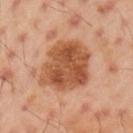workup — no biopsy performed (imaged during a skin exam)
lighting — cross-polarized
diameter — ~6 mm (longest diameter)
automated lesion analysis — a lesion area of about 27 mm², an outline eccentricity of about 0.45 (0 = round, 1 = elongated), and a shape-asymmetry score of about 0.2 (0 = symmetric); a mean CIELAB color near L≈52 a*≈25 b*≈35, roughly 13 lightness units darker than nearby skin, and a normalized border contrast of about 9.5; a within-lesion color-variation index near 5.5/10 and peripheral color asymmetry of about 2; a lesion-detection confidence of about 100/100
acquisition — 15 mm crop, total-body photography
subject — male, aged 43–47
anatomic site — the left upper arm